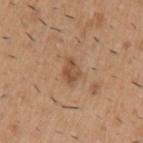Notes:
• biopsy status: catalogued during a skin exam; not biopsied
• automated metrics: a footprint of about 4 mm², an outline eccentricity of about 0.75 (0 = round, 1 = elongated), and a symmetry-axis asymmetry near 0.3; a border-irregularity index near 3/10, a within-lesion color-variation index near 3/10, and radial color variation of about 1; lesion-presence confidence of about 100/100
• subject: male, approximately 40 years of age
• imaging modality: ~15 mm tile from a whole-body skin photo
• anatomic site: the left upper arm
• tile lighting: white-light illumination